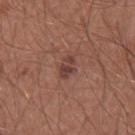Clinical impression: The lesion was photographed on a routine skin check and not biopsied; there is no pathology result. Background: Approximately 2.5 mm at its widest. Automated image analysis of the tile measured a footprint of about 3.5 mm², an eccentricity of roughly 0.8, and a shape-asymmetry score of about 0.35 (0 = symmetric). The analysis additionally found a border-irregularity rating of about 4/10. A male patient aged 28–32. On the right forearm. A 15 mm close-up extracted from a 3D total-body photography capture. Captured under white-light illumination.